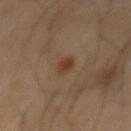notes: total-body-photography surveillance lesion; no biopsy
acquisition: 15 mm crop, total-body photography
lesion size: about 2 mm
lighting: cross-polarized illumination
TBP lesion metrics: a lesion color around L≈36 a*≈18 b*≈29 in CIELAB and a normalized lesion–skin contrast near 8.5
subject: male, aged 68–72
anatomic site: the mid back